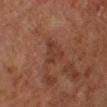{"patient": {"sex": "male", "age_approx": 80}, "image": {"source": "total-body photography crop", "field_of_view_mm": 15}, "site": "leg"}